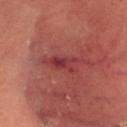Captured during whole-body skin photography for melanoma surveillance; the lesion was not biopsied. The patient is a male aged approximately 65. The recorded lesion diameter is about 4 mm. A close-up tile cropped from a whole-body skin photograph, about 15 mm across. The tile uses cross-polarized illumination. On the head or neck.